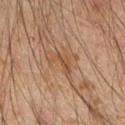notes=no biopsy performed (imaged during a skin exam) | anatomic site=the right upper arm | subject=male, aged 48–52 | image=~15 mm tile from a whole-body skin photo.